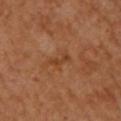workup=catalogued during a skin exam; not biopsied | image=~15 mm tile from a whole-body skin photo | location=the upper back | diameter=about 3 mm | subject=female, about 60 years old | tile lighting=cross-polarized.